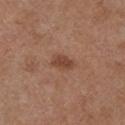{"biopsy_status": "not biopsied; imaged during a skin examination", "lesion_size": {"long_diameter_mm_approx": 3.0}, "patient": {"sex": "male", "age_approx": 55}, "lighting": "white-light", "image": {"source": "total-body photography crop", "field_of_view_mm": 15}, "site": "right upper arm", "automated_metrics": {"area_mm2_approx": 4.5, "eccentricity": 0.8, "shape_asymmetry": 0.15, "lesion_detection_confidence_0_100": 100}}